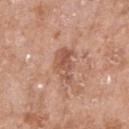Findings:
• biopsy status — total-body-photography surveillance lesion; no biopsy
• site — the right forearm
• acquisition — 15 mm crop, total-body photography
• subject — female, aged 73–77
• illumination — white-light